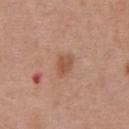<lesion>
  <biopsy_status>not biopsied; imaged during a skin examination</biopsy_status>
  <patient>
    <sex>male</sex>
    <age_approx>70</age_approx>
  </patient>
  <lighting>white-light</lighting>
  <site>front of the torso</site>
  <automated_metrics>
    <shape_asymmetry>0.25</shape_asymmetry>
    <border_irregularity_0_10>2.5</border_irregularity_0_10>
    <color_variation_0_10>2.0</color_variation_0_10>
    <peripheral_color_asymmetry>0.5</peripheral_color_asymmetry>
    <nevus_likeness_0_100>75</nevus_likeness_0_100>
    <lesion_detection_confidence_0_100>100</lesion_detection_confidence_0_100>
  </automated_metrics>
  <image>
    <source>total-body photography crop</source>
    <field_of_view_mm>15</field_of_view_mm>
  </image>
  <lesion_size>
    <long_diameter_mm_approx>2.5</long_diameter_mm_approx>
  </lesion_size>
</lesion>